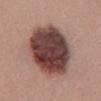{"biopsy_status": "not biopsied; imaged during a skin examination", "site": "mid back", "image": {"source": "total-body photography crop", "field_of_view_mm": 15}, "patient": {"sex": "female", "age_approx": 25}}